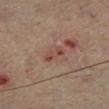Imaged during a routine full-body skin examination; the lesion was not biopsied and no histopathology is available. The lesion is on the left lower leg. The lesion-visualizer software estimated a symmetry-axis asymmetry near 0.3. And it measured a lesion color around L≈46 a*≈22 b*≈26 in CIELAB, roughly 8 lightness units darker than nearby skin, and a lesion-to-skin contrast of about 6.5 (normalized; higher = more distinct). It also reported a border-irregularity index near 3.5/10, internal color variation of about 0 on a 0–10 scale, and radial color variation of about 0. The software also gave an automated nevus-likeness rating near 0 out of 100 and a detector confidence of about 100 out of 100 that the crop contains a lesion. A 15 mm crop from a total-body photograph taken for skin-cancer surveillance. A male patient aged 63 to 67. Imaged with cross-polarized lighting. Measured at roughly 3 mm in maximum diameter.